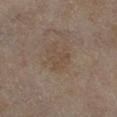Q: Was this lesion biopsied?
A: catalogued during a skin exam; not biopsied
Q: What are the patient's age and sex?
A: female, aged 58 to 62
Q: What kind of image is this?
A: total-body-photography crop, ~15 mm field of view
Q: Automated lesion metrics?
A: a border-irregularity rating of about 5.5/10 and a peripheral color-asymmetry measure near 0.5
Q: What is the anatomic site?
A: the right lower leg